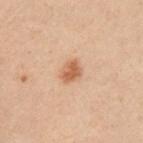Q: Patient demographics?
A: female, aged 28 to 32
Q: What is the anatomic site?
A: the left arm
Q: How was this image acquired?
A: ~15 mm tile from a whole-body skin photo